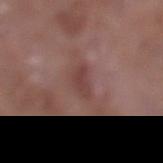Impression:
Part of a total-body skin-imaging series; this lesion was reviewed on a skin check and was not flagged for biopsy.
Background:
The recorded lesion diameter is about 2.5 mm. A 15 mm close-up tile from a total-body photography series done for melanoma screening. The tile uses white-light illumination. A male patient aged 53–57. The lesion is on the left lower leg.